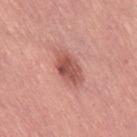follow-up — no biopsy performed (imaged during a skin exam); patient — female, aged 63 to 67; size — about 4.5 mm; illumination — white-light; image — ~15 mm tile from a whole-body skin photo; body site — the leg.